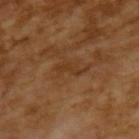Imaged during a routine full-body skin examination; the lesion was not biopsied and no histopathology is available. Longest diameter approximately 3 mm. The patient is a male aged 63–67. A region of skin cropped from a whole-body photographic capture, roughly 15 mm wide. This is a cross-polarized tile.